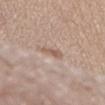Impression: This lesion was catalogued during total-body skin photography and was not selected for biopsy. Background: This is a white-light tile. An algorithmic analysis of the crop reported an area of roughly 3 mm² and two-axis asymmetry of about 0.3. The software also gave a lesion–skin lightness drop of about 8 and a normalized border contrast of about 6. The analysis additionally found an automated nevus-likeness rating near 0 out of 100 and a detector confidence of about 100 out of 100 that the crop contains a lesion. The subject is a male aged approximately 70. A 15 mm close-up tile from a total-body photography series done for melanoma screening. About 2.5 mm across. From the chest.